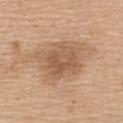workup: catalogued during a skin exam; not biopsied | patient: female, about 65 years old | lesion size: ~5 mm (longest diameter) | location: the back | image: ~15 mm tile from a whole-body skin photo.